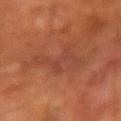Q: Is there a histopathology result?
A: catalogued during a skin exam; not biopsied
Q: What lighting was used for the tile?
A: cross-polarized illumination
Q: How was this image acquired?
A: ~15 mm tile from a whole-body skin photo
Q: Where on the body is the lesion?
A: the right forearm
Q: Automated lesion metrics?
A: an average lesion color of about L≈41 a*≈25 b*≈30 (CIELAB) and about 5 CIELAB-L* units darker than the surrounding skin; a border-irregularity rating of about 8/10
Q: Lesion size?
A: about 7 mm
Q: What are the patient's age and sex?
A: male, aged around 60